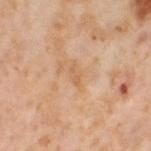Measured at roughly 2.5 mm in maximum diameter.
A female patient aged 53 to 57.
A 15 mm crop from a total-body photograph taken for skin-cancer surveillance.
Automated tile analysis of the lesion measured a detector confidence of about 100 out of 100 that the crop contains a lesion.
The lesion is located on the right thigh.